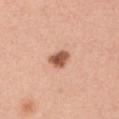Notes:
– follow-up: catalogued during a skin exam; not biopsied
– tile lighting: white-light
– image: total-body-photography crop, ~15 mm field of view
– location: the left upper arm
– patient: male, approximately 60 years of age